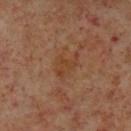Case summary:
– biopsy status — catalogued during a skin exam; not biopsied
– image — ~15 mm crop, total-body skin-cancer survey
– subject — male, about 60 years old
– anatomic site — the left lower leg